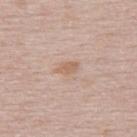biopsy status: no biopsy performed (imaged during a skin exam); site: the back; imaging modality: 15 mm crop, total-body photography; subject: male, aged around 50.